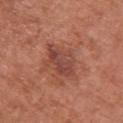Clinical impression:
Recorded during total-body skin imaging; not selected for excision or biopsy.
Background:
The patient is a female aged approximately 65. The tile uses white-light illumination. Measured at roughly 4.5 mm in maximum diameter. The lesion is located on the upper back. A 15 mm crop from a total-body photograph taken for skin-cancer surveillance.